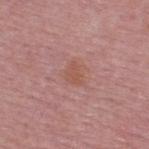Findings:
– site — the upper back
– tile lighting — white-light illumination
– patient — male, about 50 years old
– image source — ~15 mm crop, total-body skin-cancer survey
– lesion diameter — about 2.5 mm